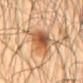Assessment: The lesion was tiled from a total-body skin photograph and was not biopsied. Acquisition and patient details: The patient is a male aged around 65. Captured under cross-polarized illumination. Located on the mid back. About 6 mm across. A 15 mm close-up extracted from a 3D total-body photography capture.